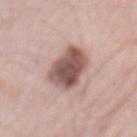Findings:
• biopsy status: no biopsy performed (imaged during a skin exam)
• subject: male, in their mid- to late 60s
• image-analysis metrics: a footprint of about 16 mm², an outline eccentricity of about 0.9 (0 = round, 1 = elongated), and two-axis asymmetry of about 0.2; an average lesion color of about L≈54 a*≈19 b*≈22 (CIELAB) and a normalized border contrast of about 11; border irregularity of about 2.5 on a 0–10 scale, internal color variation of about 6.5 on a 0–10 scale, and peripheral color asymmetry of about 2
• illumination: white-light illumination
• image source: ~15 mm crop, total-body skin-cancer survey
• site: the mid back
• diameter: ≈6.5 mm